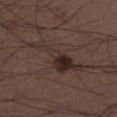<record>
<biopsy_status>not biopsied; imaged during a skin examination</biopsy_status>
<site>leg</site>
<image>
  <source>total-body photography crop</source>
  <field_of_view_mm>15</field_of_view_mm>
</image>
<patient>
  <sex>male</sex>
  <age_approx>50</age_approx>
</patient>
</record>